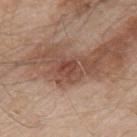The lesion was photographed on a routine skin check and not biopsied; there is no pathology result.
On the arm.
Measured at roughly 2.5 mm in maximum diameter.
Automated image analysis of the tile measured an area of roughly 5 mm², an outline eccentricity of about 0.55 (0 = round, 1 = elongated), and two-axis asymmetry of about 0.3. The software also gave a border-irregularity rating of about 3/10, a color-variation rating of about 3.5/10, and a peripheral color-asymmetry measure near 1. The software also gave an automated nevus-likeness rating near 0 out of 100 and a detector confidence of about 100 out of 100 that the crop contains a lesion.
The patient is a male roughly 55 years of age.
The tile uses white-light illumination.
A roughly 15 mm field-of-view crop from a total-body skin photograph.